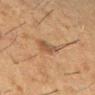Impression:
Captured during whole-body skin photography for melanoma surveillance; the lesion was not biopsied.
Background:
On the left lower leg. This image is a 15 mm lesion crop taken from a total-body photograph. A female subject about 50 years old.